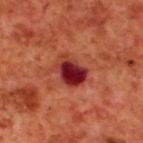Q: Was a biopsy performed?
A: catalogued during a skin exam; not biopsied
Q: Lesion size?
A: about 4 mm
Q: What lighting was used for the tile?
A: cross-polarized illumination
Q: Where on the body is the lesion?
A: the upper back
Q: How was this image acquired?
A: 15 mm crop, total-body photography
Q: Who is the patient?
A: male, roughly 70 years of age